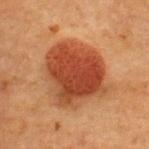Captured during whole-body skin photography for melanoma surveillance; the lesion was not biopsied. A female subject aged 38–42. Measured at roughly 7 mm in maximum diameter. Automated tile analysis of the lesion measured a footprint of about 35 mm², an outline eccentricity of about 0.45 (0 = round, 1 = elongated), and a shape-asymmetry score of about 0.25 (0 = symmetric). And it measured an average lesion color of about L≈40 a*≈27 b*≈34 (CIELAB), a lesion–skin lightness drop of about 13, and a normalized border contrast of about 10. And it measured an automated nevus-likeness rating near 100 out of 100 and a detector confidence of about 100 out of 100 that the crop contains a lesion. A close-up tile cropped from a whole-body skin photograph, about 15 mm across. The lesion is located on the upper back. Captured under cross-polarized illumination.